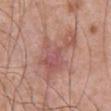Q: Was this lesion biopsied?
A: catalogued during a skin exam; not biopsied
Q: Lesion location?
A: the abdomen
Q: How large is the lesion?
A: ≈8 mm
Q: Who is the patient?
A: male, about 70 years old
Q: How was this image acquired?
A: ~15 mm crop, total-body skin-cancer survey